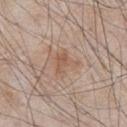Q: Was this lesion biopsied?
A: no biopsy performed (imaged during a skin exam)
Q: What lighting was used for the tile?
A: white-light
Q: What is the imaging modality?
A: ~15 mm tile from a whole-body skin photo
Q: Automated lesion metrics?
A: a shape-asymmetry score of about 0.45 (0 = symmetric); a border-irregularity index near 5/10, internal color variation of about 3.5 on a 0–10 scale, and radial color variation of about 1.5; lesion-presence confidence of about 100/100
Q: What is the anatomic site?
A: the chest
Q: Patient demographics?
A: male, in their mid-60s